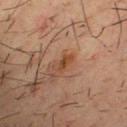Q: Is there a histopathology result?
A: total-body-photography surveillance lesion; no biopsy
Q: How large is the lesion?
A: ~2.5 mm (longest diameter)
Q: How was this image acquired?
A: 15 mm crop, total-body photography
Q: What are the patient's age and sex?
A: male, roughly 35 years of age
Q: Lesion location?
A: the chest
Q: How was the tile lit?
A: cross-polarized